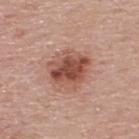An algorithmic analysis of the crop reported a lesion color around L≈51 a*≈24 b*≈28 in CIELAB, roughly 13 lightness units darker than nearby skin, and a normalized border contrast of about 9. The software also gave an automated nevus-likeness rating near 75 out of 100. The recorded lesion diameter is about 5 mm. Captured under white-light illumination. A male subject approximately 55 years of age. From the upper back. A 15 mm crop from a total-body photograph taken for skin-cancer surveillance.